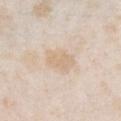Notes:
- follow-up — imaged on a skin check; not biopsied
- body site — the chest
- image — ~15 mm tile from a whole-body skin photo
- illumination — white-light illumination
- automated metrics — a footprint of about 9.5 mm², an eccentricity of roughly 0.65, and a shape-asymmetry score of about 0.2 (0 = symmetric); about 6 CIELAB-L* units darker than the surrounding skin and a lesion-to-skin contrast of about 5 (normalized; higher = more distinct)
- size — ≈4 mm
- patient — female, about 25 years old